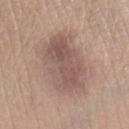{"biopsy_status": "not biopsied; imaged during a skin examination", "automated_metrics": {"area_mm2_approx": 25.0, "cielab_L": 54, "cielab_a": 17, "cielab_b": 22, "vs_skin_darker_L": 10.0, "vs_skin_contrast_norm": 7.5, "nevus_likeness_0_100": 10, "lesion_detection_confidence_0_100": 100}, "patient": {"sex": "female", "age_approx": 60}, "lesion_size": {"long_diameter_mm_approx": 7.5}, "site": "right lower leg", "lighting": "white-light", "image": {"source": "total-body photography crop", "field_of_view_mm": 15}}